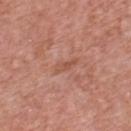* size · ≈3 mm
* automated metrics · about 6 CIELAB-L* units darker than the surrounding skin and a lesion-to-skin contrast of about 5 (normalized; higher = more distinct); border irregularity of about 3 on a 0–10 scale, internal color variation of about 0.5 on a 0–10 scale, and a peripheral color-asymmetry measure near 0
* lighting · white-light
* location · the front of the torso
* imaging modality · total-body-photography crop, ~15 mm field of view
* patient · male, aged around 70